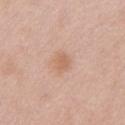The lesion was photographed on a routine skin check and not biopsied; there is no pathology result. The tile uses white-light illumination. A female patient, aged approximately 40. This image is a 15 mm lesion crop taken from a total-body photograph. Automated tile analysis of the lesion measured a border-irregularity rating of about 1.5/10, a color-variation rating of about 1.5/10, and peripheral color asymmetry of about 0.5. It also reported a nevus-likeness score of about 20/100 and a lesion-detection confidence of about 100/100. Located on the chest.